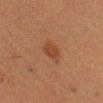Impression: The lesion was photographed on a routine skin check and not biopsied; there is no pathology result. Image and clinical context: Cropped from a whole-body photographic skin survey; the tile spans about 15 mm. A male patient, aged 38 to 42. The lesion is on the head or neck. Longest diameter approximately 2.5 mm. The tile uses cross-polarized illumination.